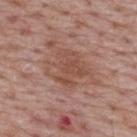biopsy status — imaged on a skin check; not biopsied
patient — male, in their mid- to late 60s
imaging modality — ~15 mm tile from a whole-body skin photo
site — the upper back